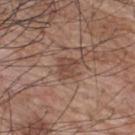No biopsy was performed on this lesion — it was imaged during a full skin examination and was not determined to be concerning.
Imaged with white-light lighting.
A region of skin cropped from a whole-body photographic capture, roughly 15 mm wide.
The lesion is on the upper back.
Approximately 3 mm at its widest.
A male patient, aged 68 to 72.
Automated image analysis of the tile measured a footprint of about 5.5 mm² and a shape-asymmetry score of about 0.3 (0 = symmetric). The software also gave about 8 CIELAB-L* units darker than the surrounding skin and a normalized lesion–skin contrast near 6.5.